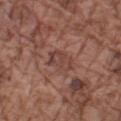Q: Was a biopsy performed?
A: catalogued during a skin exam; not biopsied
Q: Lesion location?
A: the left forearm
Q: What kind of image is this?
A: total-body-photography crop, ~15 mm field of view
Q: What did automated image analysis measure?
A: a border-irregularity index near 3.5/10, internal color variation of about 2.5 on a 0–10 scale, and radial color variation of about 1; lesion-presence confidence of about 85/100
Q: Who is the patient?
A: male, in their mid- to late 70s
Q: Illumination type?
A: white-light
Q: What is the lesion's diameter?
A: ~3 mm (longest diameter)